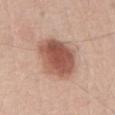No biopsy was performed on this lesion — it was imaged during a full skin examination and was not determined to be concerning.
A male patient roughly 70 years of age.
A 15 mm close-up extracted from a 3D total-body photography capture.
Located on the abdomen.